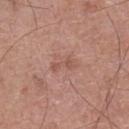Recorded during total-body skin imaging; not selected for excision or biopsy.